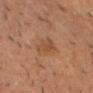Assessment:
This lesion was catalogued during total-body skin photography and was not selected for biopsy.
Acquisition and patient details:
A male patient, in their mid- to late 60s. The recorded lesion diameter is about 4 mm. Cropped from a whole-body photographic skin survey; the tile spans about 15 mm. From the upper back.